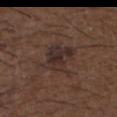Captured during whole-body skin photography for melanoma surveillance; the lesion was not biopsied. Longest diameter approximately 4 mm. An algorithmic analysis of the crop reported an area of roughly 11 mm², an eccentricity of roughly 0.5, and a symmetry-axis asymmetry near 0.3. The analysis additionally found a border-irregularity rating of about 3.5/10, a within-lesion color-variation index near 4.5/10, and radial color variation of about 1.5. The software also gave lesion-presence confidence of about 100/100. On the upper back. A roughly 15 mm field-of-view crop from a total-body skin photograph. The patient is a male aged 48–52.